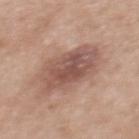workup=no biopsy performed (imaged during a skin exam); image=15 mm crop, total-body photography; subject=female, about 50 years old; lesion diameter=~6.5 mm (longest diameter); lighting=white-light illumination; site=the upper back.